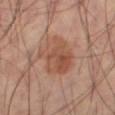follow-up — no biopsy performed (imaged during a skin exam) | lighting — cross-polarized | site — the right thigh | subject — male, aged 58 to 62 | lesion diameter — about 5 mm | image source — ~15 mm tile from a whole-body skin photo | image-analysis metrics — an average lesion color of about L≈49 a*≈21 b*≈29 (CIELAB), a lesion–skin lightness drop of about 9, and a lesion-to-skin contrast of about 7 (normalized; higher = more distinct); a nevus-likeness score of about 40/100 and lesion-presence confidence of about 100/100.